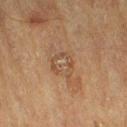Impression:
The lesion was photographed on a routine skin check and not biopsied; there is no pathology result.
Clinical summary:
Automated tile analysis of the lesion measured a lesion area of about 4.5 mm², a shape eccentricity near 0.5, and a shape-asymmetry score of about 0.45 (0 = symmetric). The software also gave a mean CIELAB color near L≈42 a*≈16 b*≈29, about 6 CIELAB-L* units darker than the surrounding skin, and a normalized lesion–skin contrast near 5. The software also gave a border-irregularity rating of about 8.5/10, a within-lesion color-variation index near 0/10, and a peripheral color-asymmetry measure near 0. The lesion is on the left lower leg. A male subject aged 83 or older. A region of skin cropped from a whole-body photographic capture, roughly 15 mm wide. The recorded lesion diameter is about 3 mm. This is a cross-polarized tile.